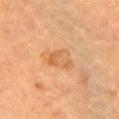workup: imaged on a skin check; not biopsied
patient: female, aged 68–72
diameter: ≈4 mm
location: the chest
image: ~15 mm crop, total-body skin-cancer survey
image-analysis metrics: a border-irregularity index near 3/10 and peripheral color asymmetry of about 1.5